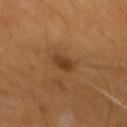Q: Was a biopsy performed?
A: no biopsy performed (imaged during a skin exam)
Q: Who is the patient?
A: male, about 60 years old
Q: Where on the body is the lesion?
A: the back
Q: What kind of image is this?
A: ~15 mm tile from a whole-body skin photo
Q: How was the tile lit?
A: cross-polarized